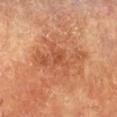Impression: This lesion was catalogued during total-body skin photography and was not selected for biopsy. Context: The lesion is located on the chest. About 6.5 mm across. Automated tile analysis of the lesion measured a shape eccentricity near 0.85. The analysis additionally found a nevus-likeness score of about 0/100 and a lesion-detection confidence of about 100/100. This image is a 15 mm lesion crop taken from a total-body photograph. The patient is a female aged 78–82. This is a cross-polarized tile.